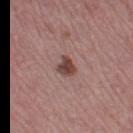Imaged during a routine full-body skin examination; the lesion was not biopsied and no histopathology is available. The lesion is on the left thigh. Approximately 2.5 mm at its widest. A female subject, approximately 45 years of age. The total-body-photography lesion software estimated a mean CIELAB color near L≈44 a*≈20 b*≈22, a lesion–skin lightness drop of about 13, and a lesion-to-skin contrast of about 10 (normalized; higher = more distinct). The analysis additionally found a within-lesion color-variation index near 3.5/10. Cropped from a total-body skin-imaging series; the visible field is about 15 mm. This is a white-light tile.